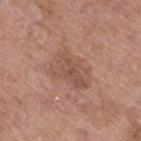Impression:
No biopsy was performed on this lesion — it was imaged during a full skin examination and was not determined to be concerning.
Image and clinical context:
An algorithmic analysis of the crop reported a footprint of about 13 mm², an eccentricity of roughly 0.75, and two-axis asymmetry of about 0.25. The analysis additionally found a border-irregularity index near 3.5/10, internal color variation of about 3 on a 0–10 scale, and peripheral color asymmetry of about 1.5. The patient is a female about 85 years old or older. About 5 mm across. A roughly 15 mm field-of-view crop from a total-body skin photograph. On the left lower leg.